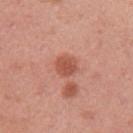<case>
<biopsy_status>not biopsied; imaged during a skin examination</biopsy_status>
<site>left upper arm</site>
<lesion_size>
  <long_diameter_mm_approx>3.0</long_diameter_mm_approx>
</lesion_size>
<patient>
  <sex>female</sex>
  <age_approx>50</age_approx>
</patient>
<image>
  <source>total-body photography crop</source>
  <field_of_view_mm>15</field_of_view_mm>
</image>
</case>